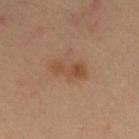Part of a total-body skin-imaging series; this lesion was reviewed on a skin check and was not flagged for biopsy.
A male patient aged around 55.
A close-up tile cropped from a whole-body skin photograph, about 15 mm across.
On the front of the torso.
The recorded lesion diameter is about 4 mm.
Imaged with cross-polarized lighting.
An algorithmic analysis of the crop reported a lesion area of about 6.5 mm² and a shape-asymmetry score of about 0.3 (0 = symmetric). The analysis additionally found a lesion color around L≈38 a*≈17 b*≈27 in CIELAB, a lesion–skin lightness drop of about 6, and a lesion-to-skin contrast of about 6.5 (normalized; higher = more distinct). It also reported peripheral color asymmetry of about 1. It also reported an automated nevus-likeness rating near 20 out of 100.